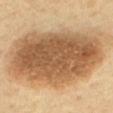  biopsy_status: not biopsied; imaged during a skin examination
  automated_metrics:
    eccentricity: 0.85
    shape_asymmetry: 0.2
    vs_skin_darker_L: 16.0
    vs_skin_contrast_norm: 10.0
    nevus_likeness_0_100: 100
  patient:
    age_approx: 70
  lesion_size:
    long_diameter_mm_approx: 15.0
  site: mid back
  image:
    source: total-body photography crop
    field_of_view_mm: 15